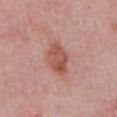follow-up = imaged on a skin check; not biopsied.